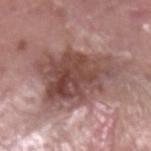The lesion was photographed on a routine skin check and not biopsied; there is no pathology result.